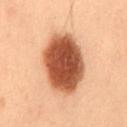This lesion was catalogued during total-body skin photography and was not selected for biopsy.
A male subject aged 53–57.
A 15 mm close-up tile from a total-body photography series done for melanoma screening.
The lesion is located on the mid back.
Captured under cross-polarized illumination.